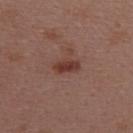Assessment:
The lesion was tiled from a total-body skin photograph and was not biopsied.
Clinical summary:
The tile uses white-light illumination. Cropped from a whole-body photographic skin survey; the tile spans about 15 mm. A female patient, in their mid-40s. The recorded lesion diameter is about 3 mm. From the upper back.